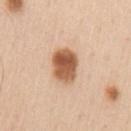{
  "lesion_size": {
    "long_diameter_mm_approx": 4.0
  },
  "patient": {
    "sex": "male",
    "age_approx": 60
  },
  "lighting": "white-light",
  "site": "arm",
  "image": {
    "source": "total-body photography crop",
    "field_of_view_mm": 15
  }
}